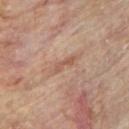Impression: This lesion was catalogued during total-body skin photography and was not selected for biopsy. Clinical summary: Longest diameter approximately 3 mm. This is a cross-polarized tile. From the upper back. Automated tile analysis of the lesion measured a mean CIELAB color near L≈52 a*≈21 b*≈29, a lesion–skin lightness drop of about 8, and a normalized border contrast of about 6. The analysis additionally found a lesion-detection confidence of about 55/100. This image is a 15 mm lesion crop taken from a total-body photograph. The subject is a male aged around 85 or older.